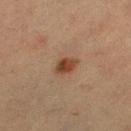Imaged during a routine full-body skin examination; the lesion was not biopsied and no histopathology is available.
Imaged with cross-polarized lighting.
An algorithmic analysis of the crop reported an average lesion color of about L≈33 a*≈18 b*≈26 (CIELAB), roughly 10 lightness units darker than nearby skin, and a lesion-to-skin contrast of about 9.5 (normalized; higher = more distinct). The software also gave a nevus-likeness score of about 100/100 and a detector confidence of about 100 out of 100 that the crop contains a lesion.
A 15 mm close-up tile from a total-body photography series done for melanoma screening.
A female subject roughly 55 years of age.
Approximately 2.5 mm at its widest.
The lesion is on the leg.